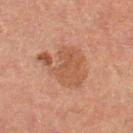The lesion was tiled from a total-body skin photograph and was not biopsied.
On the left thigh.
This image is a 15 mm lesion crop taken from a total-body photograph.
The tile uses cross-polarized illumination.
About 6 mm across.
Automated tile analysis of the lesion measured an average lesion color of about L≈43 a*≈19 b*≈28 (CIELAB), roughly 7 lightness units darker than nearby skin, and a lesion-to-skin contrast of about 6.5 (normalized; higher = more distinct). It also reported a border-irregularity rating of about 5.5/10 and peripheral color asymmetry of about 0.5. The analysis additionally found a classifier nevus-likeness of about 0/100 and lesion-presence confidence of about 100/100.
The patient is a female approximately 60 years of age.